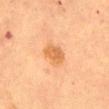Assessment:
The lesion was tiled from a total-body skin photograph and was not biopsied.
Context:
From the abdomen. The tile uses cross-polarized illumination. About 2.5 mm across. The patient is a female approximately 60 years of age. A lesion tile, about 15 mm wide, cut from a 3D total-body photograph. The total-body-photography lesion software estimated an outline eccentricity of about 0.55 (0 = round, 1 = elongated) and two-axis asymmetry of about 0.3. It also reported a border-irregularity rating of about 2.5/10 and a within-lesion color-variation index near 2.5/10.